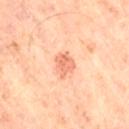This is a cross-polarized tile. A male subject, in their mid- to late 60s. Cropped from a total-body skin-imaging series; the visible field is about 15 mm. The total-body-photography lesion software estimated a lesion area of about 5 mm², an eccentricity of roughly 0.65, and a shape-asymmetry score of about 0.25 (0 = symmetric). The analysis additionally found a classifier nevus-likeness of about 20/100 and a lesion-detection confidence of about 100/100. Longest diameter approximately 3 mm. On the right thigh.